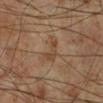Clinical impression:
No biopsy was performed on this lesion — it was imaged during a full skin examination and was not determined to be concerning.
Clinical summary:
The lesion is on the leg. The lesion-visualizer software estimated a footprint of about 4 mm², a shape eccentricity near 0.8, and two-axis asymmetry of about 0.55. The software also gave an average lesion color of about L≈39 a*≈15 b*≈28 (CIELAB), roughly 6 lightness units darker than nearby skin, and a normalized border contrast of about 6. And it measured a border-irregularity rating of about 5.5/10 and a within-lesion color-variation index near 2/10. It also reported a nevus-likeness score of about 0/100 and a lesion-detection confidence of about 100/100. A 15 mm close-up extracted from a 3D total-body photography capture. The tile uses cross-polarized illumination. The patient is a male approximately 60 years of age.